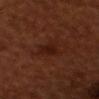A close-up tile cropped from a whole-body skin photograph, about 15 mm across. Located on the head or neck. The patient is a male about 55 years old.